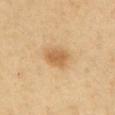<case>
<biopsy_status>not biopsied; imaged during a skin examination</biopsy_status>
<image>
  <source>total-body photography crop</source>
  <field_of_view_mm>15</field_of_view_mm>
</image>
<automated_metrics>
  <area_mm2_approx>6.0</area_mm2_approx>
  <eccentricity>0.75</eccentricity>
  <shape_asymmetry>0.25</shape_asymmetry>
  <cielab_L>56</cielab_L>
  <cielab_a>18</cielab_a>
  <cielab_b>38</cielab_b>
  <vs_skin_darker_L>10.0</vs_skin_darker_L>
  <vs_skin_contrast_norm>7.0</vs_skin_contrast_norm>
  <border_irregularity_0_10>2.0</border_irregularity_0_10>
  <color_variation_0_10>2.5</color_variation_0_10>
  <nevus_likeness_0_100>90</nevus_likeness_0_100>
</automated_metrics>
<lesion_size>
  <long_diameter_mm_approx>3.5</long_diameter_mm_approx>
</lesion_size>
<site>upper back</site>
<patient>
  <sex>male</sex>
  <age_approx>55</age_approx>
</patient>
</case>